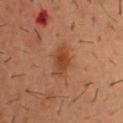notes — imaged on a skin check; not biopsied
anatomic site — the chest
automated lesion analysis — an area of roughly 7.5 mm², an eccentricity of roughly 0.75, and a shape-asymmetry score of about 0.25 (0 = symmetric); a lesion color around L≈38 a*≈21 b*≈31 in CIELAB, about 7 CIELAB-L* units darker than the surrounding skin, and a lesion-to-skin contrast of about 7 (normalized; higher = more distinct)
patient — male, approximately 55 years of age
illumination — cross-polarized illumination
imaging modality — 15 mm crop, total-body photography
size — about 4 mm